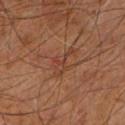| feature | finding |
|---|---|
| biopsy status | imaged on a skin check; not biopsied |
| location | the right leg |
| subject | male, in their 60s |
| illumination | cross-polarized |
| acquisition | total-body-photography crop, ~15 mm field of view |